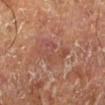follow-up=catalogued during a skin exam; not biopsied | diameter=~5.5 mm (longest diameter) | tile lighting=cross-polarized illumination | subject=male, roughly 75 years of age | site=the left lower leg | image=total-body-photography crop, ~15 mm field of view.